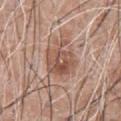Case summary:
• follow-up — no biopsy performed (imaged during a skin exam)
• automated lesion analysis — a footprint of about 12 mm²; border irregularity of about 4 on a 0–10 scale, internal color variation of about 6.5 on a 0–10 scale, and peripheral color asymmetry of about 2.5
• anatomic site — the abdomen
• acquisition — ~15 mm crop, total-body skin-cancer survey
• subject — male, aged approximately 60